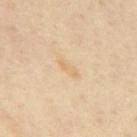| field | value |
|---|---|
| workup | imaged on a skin check; not biopsied |
| acquisition | ~15 mm crop, total-body skin-cancer survey |
| illumination | cross-polarized |
| subject | male, roughly 65 years of age |
| lesion size | ≈3 mm |
| TBP lesion metrics | a footprint of about 3.5 mm², an eccentricity of roughly 0.95, and a shape-asymmetry score of about 0.35 (0 = symmetric); border irregularity of about 3.5 on a 0–10 scale; a classifier nevus-likeness of about 0/100 |
| location | the mid back |